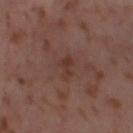follow-up: imaged on a skin check; not biopsied | subject: female, aged 53 to 57 | acquisition: total-body-photography crop, ~15 mm field of view | anatomic site: the left thigh | size: ~3 mm (longest diameter) | image-analysis metrics: a lesion–skin lightness drop of about 6 and a lesion-to-skin contrast of about 5.5 (normalized; higher = more distinct); border irregularity of about 5 on a 0–10 scale, a color-variation rating of about 0.5/10, and a peripheral color-asymmetry measure near 0.